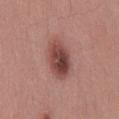Assessment:
This lesion was catalogued during total-body skin photography and was not selected for biopsy.
Background:
A roughly 15 mm field-of-view crop from a total-body skin photograph. The subject is a male aged 28–32. On the mid back.